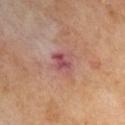follow-up — catalogued during a skin exam; not biopsied
subject — male, aged 68–72
image source — ~15 mm crop, total-body skin-cancer survey
lesion size — ≈3 mm
illumination — cross-polarized illumination
location — the arm